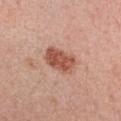Clinical impression:
The lesion was photographed on a routine skin check and not biopsied; there is no pathology result.
Acquisition and patient details:
The patient is a female approximately 45 years of age. A close-up tile cropped from a whole-body skin photograph, about 15 mm across. The lesion is on the chest.